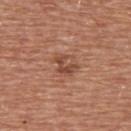automated_metrics:
  color_variation_0_10: 1.0
  peripheral_color_asymmetry: 0.5
  nevus_likeness_0_100: 5
  lesion_detection_confidence_0_100: 100
lighting: white-light
site: upper back
lesion_size:
  long_diameter_mm_approx: 3.0
patient:
  sex: male
  age_approx: 65
image:
  source: total-body photography crop
  field_of_view_mm: 15